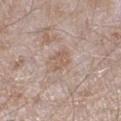Q: Was a biopsy performed?
A: no biopsy performed (imaged during a skin exam)
Q: Where on the body is the lesion?
A: the right lower leg
Q: What is the imaging modality?
A: ~15 mm crop, total-body skin-cancer survey
Q: Patient demographics?
A: male, in their mid- to late 40s